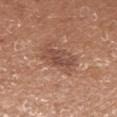<lesion>
<biopsy_status>not biopsied; imaged during a skin examination</biopsy_status>
<site>arm</site>
<patient>
  <sex>female</sex>
  <age_approx>35</age_approx>
</patient>
<image>
  <source>total-body photography crop</source>
  <field_of_view_mm>15</field_of_view_mm>
</image>
</lesion>